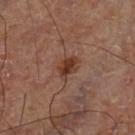The lesion was tiled from a total-body skin photograph and was not biopsied.
On the left lower leg.
Cropped from a whole-body photographic skin survey; the tile spans about 15 mm.
The tile uses cross-polarized illumination.
About 3 mm across.
Automated tile analysis of the lesion measured a footprint of about 5 mm², an outline eccentricity of about 0.7 (0 = round, 1 = elongated), and a symmetry-axis asymmetry near 0.3.